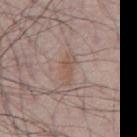The patient is a male roughly 55 years of age. Imaged with white-light lighting. A lesion tile, about 15 mm wide, cut from a 3D total-body photograph. From the abdomen. About 3.5 mm across.